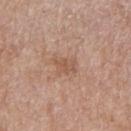follow-up=total-body-photography surveillance lesion; no biopsy | size=about 2.5 mm | subject=male, aged 68–72 | image source=~15 mm crop, total-body skin-cancer survey | anatomic site=the left upper arm | illumination=white-light illumination.